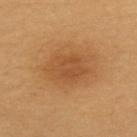{"biopsy_status": "not biopsied; imaged during a skin examination", "lighting": "cross-polarized", "patient": {"sex": "female", "age_approx": 30}, "image": {"source": "total-body photography crop", "field_of_view_mm": 15}, "site": "upper back", "automated_metrics": {"area_mm2_approx": 13.0, "eccentricity": 0.7, "shape_asymmetry": 0.2, "cielab_L": 52, "cielab_a": 23, "cielab_b": 41, "vs_skin_darker_L": 8.0, "border_irregularity_0_10": 2.5, "color_variation_0_10": 3.0, "peripheral_color_asymmetry": 1.0, "nevus_likeness_0_100": 95, "lesion_detection_confidence_0_100": 100}}